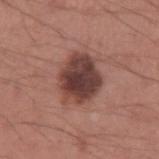A male subject, aged 33 to 37. This is a white-light tile. The lesion is on the left upper arm. A lesion tile, about 15 mm wide, cut from a 3D total-body photograph. Longest diameter approximately 5.5 mm.